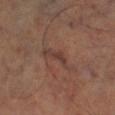Part of a total-body skin-imaging series; this lesion was reviewed on a skin check and was not flagged for biopsy. Cropped from a total-body skin-imaging series; the visible field is about 15 mm. Captured under cross-polarized illumination. The lesion's longest dimension is about 5 mm. A male subject, aged approximately 60. Automated image analysis of the tile measured a lesion area of about 6 mm², an eccentricity of roughly 0.95, and two-axis asymmetry of about 0.55. And it measured a lesion color around L≈37 a*≈19 b*≈22 in CIELAB and roughly 7 lightness units darker than nearby skin. The lesion is located on the left lower leg.